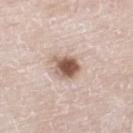Part of a total-body skin-imaging series; this lesion was reviewed on a skin check and was not flagged for biopsy. A male patient, approximately 80 years of age. On the right lower leg. A 15 mm close-up tile from a total-body photography series done for melanoma screening. About 3.5 mm across. Automated image analysis of the tile measured a lesion area of about 7.5 mm², an outline eccentricity of about 0.7 (0 = round, 1 = elongated), and a symmetry-axis asymmetry near 0.25. The analysis additionally found border irregularity of about 3 on a 0–10 scale and a color-variation rating of about 5.5/10. Captured under white-light illumination.